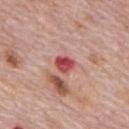Impression: Recorded during total-body skin imaging; not selected for excision or biopsy. Image and clinical context: The lesion is located on the mid back. A male patient about 75 years old. A 15 mm crop from a total-body photograph taken for skin-cancer surveillance. This is a white-light tile. The lesion's longest dimension is about 2.5 mm. Automated tile analysis of the lesion measured an automated nevus-likeness rating near 0 out of 100 and lesion-presence confidence of about 100/100.